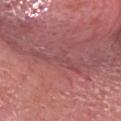This is a white-light tile. A female patient aged 58–62. Cropped from a whole-body photographic skin survey; the tile spans about 15 mm. The recorded lesion diameter is about 6.5 mm. On the head or neck.